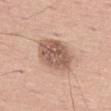Impression:
Imaged during a routine full-body skin examination; the lesion was not biopsied and no histopathology is available.
Clinical summary:
The subject is a male in their mid-70s. This is a white-light tile. Longest diameter approximately 5.5 mm. On the left thigh. A region of skin cropped from a whole-body photographic capture, roughly 15 mm wide.